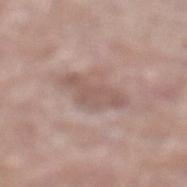Captured during whole-body skin photography for melanoma surveillance; the lesion was not biopsied.
Automated image analysis of the tile measured a border-irregularity rating of about 5/10 and peripheral color asymmetry of about 0.5.
Cropped from a total-body skin-imaging series; the visible field is about 15 mm.
The lesion is on the left lower leg.
A male subject about 70 years old.
About 5.5 mm across.